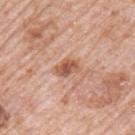The lesion was photographed on a routine skin check and not biopsied; there is no pathology result. A 15 mm crop from a total-body photograph taken for skin-cancer surveillance. A male patient, aged 78 to 82. The lesion is located on the left upper arm.